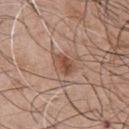Captured during whole-body skin photography for melanoma surveillance; the lesion was not biopsied.
A male patient aged 43 to 47.
Cropped from a whole-body photographic skin survey; the tile spans about 15 mm.
From the chest.